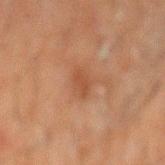Clinical impression: The lesion was photographed on a routine skin check and not biopsied; there is no pathology result. Image and clinical context: A male subject in their 60s. From the mid back. The tile uses cross-polarized illumination. The lesion's longest dimension is about 3 mm. A 15 mm close-up extracted from a 3D total-body photography capture.